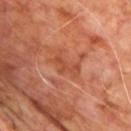Q: Was this lesion biopsied?
A: total-body-photography surveillance lesion; no biopsy
Q: Lesion location?
A: the upper back
Q: How was this image acquired?
A: 15 mm crop, total-body photography
Q: Lesion size?
A: ~3 mm (longest diameter)
Q: Who is the patient?
A: male, in their 60s